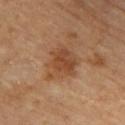Assessment:
Part of a total-body skin-imaging series; this lesion was reviewed on a skin check and was not flagged for biopsy.